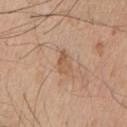This lesion was catalogued during total-body skin photography and was not selected for biopsy.
A male patient, approximately 60 years of age.
From the chest.
A close-up tile cropped from a whole-body skin photograph, about 15 mm across.
The lesion-visualizer software estimated a footprint of about 3.5 mm², a shape eccentricity near 0.75, and a symmetry-axis asymmetry near 0.4. It also reported an average lesion color of about L≈56 a*≈20 b*≈32 (CIELAB), roughly 8 lightness units darker than nearby skin, and a normalized lesion–skin contrast near 6.
Measured at roughly 2.5 mm in maximum diameter.